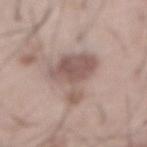{
  "biopsy_status": "not biopsied; imaged during a skin examination",
  "image": {
    "source": "total-body photography crop",
    "field_of_view_mm": 15
  },
  "lesion_size": {
    "long_diameter_mm_approx": 6.5
  },
  "site": "abdomen",
  "lighting": "white-light",
  "patient": {
    "sex": "male",
    "age_approx": 55
  }
}